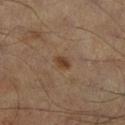Case summary:
* workup · total-body-photography surveillance lesion; no biopsy
* lighting · cross-polarized illumination
* patient · male, in their mid-50s
* automated metrics · a footprint of about 3 mm², an outline eccentricity of about 0.75 (0 = round, 1 = elongated), and a shape-asymmetry score of about 0.15 (0 = symmetric); a lesion color around L≈37 a*≈16 b*≈28 in CIELAB, about 8 CIELAB-L* units darker than the surrounding skin, and a lesion-to-skin contrast of about 7.5 (normalized; higher = more distinct); peripheral color asymmetry of about 1; an automated nevus-likeness rating near 90 out of 100 and lesion-presence confidence of about 100/100
* lesion diameter · ≈2.5 mm
* image · 15 mm crop, total-body photography
* body site · the leg